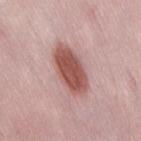• biopsy status · catalogued during a skin exam; not biopsied
• acquisition · 15 mm crop, total-body photography
• subject · female, roughly 40 years of age
• site · the right thigh
• lesion size · ≈6 mm